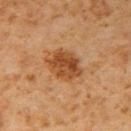Q: How was the tile lit?
A: cross-polarized
Q: Patient demographics?
A: male, about 60 years old
Q: Where on the body is the lesion?
A: the right upper arm
Q: What kind of image is this?
A: 15 mm crop, total-body photography
Q: Lesion size?
A: about 4.5 mm
Q: What did automated image analysis measure?
A: a within-lesion color-variation index near 4.5/10 and peripheral color asymmetry of about 1.5; a nevus-likeness score of about 85/100